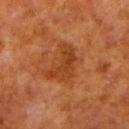Recorded during total-body skin imaging; not selected for excision or biopsy. A 15 mm crop from a total-body photograph taken for skin-cancer surveillance. Longest diameter approximately 4 mm. A male subject, aged 78–82. This is a cross-polarized tile. The lesion is located on the left lower leg.